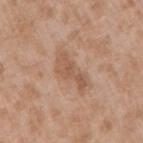Captured during whole-body skin photography for melanoma surveillance; the lesion was not biopsied. From the right upper arm. Measured at roughly 4.5 mm in maximum diameter. A lesion tile, about 15 mm wide, cut from a 3D total-body photograph. An algorithmic analysis of the crop reported a footprint of about 7 mm², an outline eccentricity of about 0.9 (0 = round, 1 = elongated), and a shape-asymmetry score of about 0.45 (0 = symmetric). It also reported a border-irregularity index near 5.5/10, a within-lesion color-variation index near 2.5/10, and peripheral color asymmetry of about 1. The analysis additionally found a classifier nevus-likeness of about 0/100 and a lesion-detection confidence of about 100/100. The tile uses white-light illumination. A male subject aged 48–52.